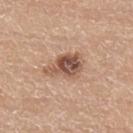{
  "biopsy_status": "not biopsied; imaged during a skin examination",
  "lighting": "white-light",
  "patient": {
    "sex": "male",
    "age_approx": 40
  },
  "site": "left lower leg",
  "lesion_size": {
    "long_diameter_mm_approx": 5.0
  },
  "image": {
    "source": "total-body photography crop",
    "field_of_view_mm": 15
  },
  "automated_metrics": {
    "area_mm2_approx": 10.0,
    "nevus_likeness_0_100": 75,
    "lesion_detection_confidence_0_100": 100
  }
}